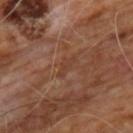Impression:
Captured during whole-body skin photography for melanoma surveillance; the lesion was not biopsied.
Context:
Located on the chest. The tile uses cross-polarized illumination. A 15 mm close-up extracted from a 3D total-body photography capture. The patient is a male in their 60s. About 3 mm across.